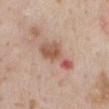Clinical impression:
Part of a total-body skin-imaging series; this lesion was reviewed on a skin check and was not flagged for biopsy.
Context:
Imaged with white-light lighting. The patient is a male about 60 years old. A lesion tile, about 15 mm wide, cut from a 3D total-body photograph. The lesion is on the back. The recorded lesion diameter is about 5.5 mm.